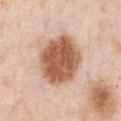  biopsy_status: not biopsied; imaged during a skin examination
  lesion_size:
    long_diameter_mm_approx: 6.5
  automated_metrics:
    cielab_L: 58
    cielab_a: 23
    cielab_b: 32
    vs_skin_darker_L: 18.0
    vs_skin_contrast_norm: 11.5
    nevus_likeness_0_100: 70
  patient:
    sex: male
    age_approx: 50
  image:
    source: total-body photography crop
    field_of_view_mm: 15
  site: chest